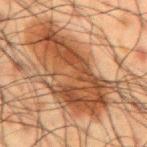Impression: Part of a total-body skin-imaging series; this lesion was reviewed on a skin check and was not flagged for biopsy. Background: On the mid back. Automated image analysis of the tile measured a footprint of about 60 mm², an eccentricity of roughly 0.9, and a shape-asymmetry score of about 0.35 (0 = symmetric). It also reported about 12 CIELAB-L* units darker than the surrounding skin and a normalized lesion–skin contrast near 9.5. The software also gave a border-irregularity index near 7/10, internal color variation of about 8 on a 0–10 scale, and a peripheral color-asymmetry measure near 3. Captured under cross-polarized illumination. A male subject aged 58 to 62. The recorded lesion diameter is about 15.5 mm. A region of skin cropped from a whole-body photographic capture, roughly 15 mm wide.